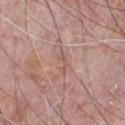| key | value |
|---|---|
| biopsy status | no biopsy performed (imaged during a skin exam) |
| image | 15 mm crop, total-body photography |
| patient | male, aged 68 to 72 |
| lesion diameter | about 2.5 mm |
| site | the chest |
| automated lesion analysis | an area of roughly 2 mm² and an outline eccentricity of about 0.95 (0 = round, 1 = elongated); a color-variation rating of about 0/10 and radial color variation of about 0 |